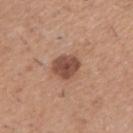follow-up: imaged on a skin check; not biopsied
acquisition: ~15 mm tile from a whole-body skin photo
subject: male, about 50 years old
image-analysis metrics: a shape eccentricity near 0.7; a lesion color around L≈48 a*≈21 b*≈28 in CIELAB, roughly 13 lightness units darker than nearby skin, and a normalized lesion–skin contrast near 9.5; internal color variation of about 2.5 on a 0–10 scale and radial color variation of about 1
illumination: white-light illumination
site: the right upper arm